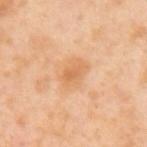Case summary:
* workup: no biopsy performed (imaged during a skin exam)
* patient: female, aged 53–57
* acquisition: 15 mm crop, total-body photography
* image-analysis metrics: a border-irregularity rating of about 2.5/10, a color-variation rating of about 3.5/10, and a peripheral color-asymmetry measure near 1; an automated nevus-likeness rating near 0 out of 100 and a lesion-detection confidence of about 100/100
* lighting: cross-polarized illumination
* site: the back